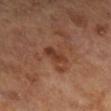This lesion was catalogued during total-body skin photography and was not selected for biopsy. The lesion is located on the right lower leg. A 15 mm crop from a total-body photograph taken for skin-cancer surveillance. A female subject, roughly 65 years of age. Imaged with cross-polarized lighting. Automated tile analysis of the lesion measured a classifier nevus-likeness of about 0/100 and a detector confidence of about 100 out of 100 that the crop contains a lesion. Longest diameter approximately 3 mm.